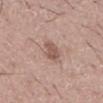Recorded during total-body skin imaging; not selected for excision or biopsy. The lesion is located on the abdomen. Longest diameter approximately 3 mm. A male patient in their mid-40s. Captured under white-light illumination. Automated tile analysis of the lesion measured a mean CIELAB color near L≈55 a*≈19 b*≈25, a lesion–skin lightness drop of about 10, and a normalized lesion–skin contrast near 7. It also reported a nevus-likeness score of about 15/100 and a detector confidence of about 100 out of 100 that the crop contains a lesion. A roughly 15 mm field-of-view crop from a total-body skin photograph.